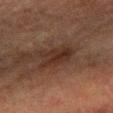notes — no biopsy performed (imaged during a skin exam)
size — about 4 mm
lighting — cross-polarized illumination
site — the right forearm
subject — female, aged 53–57
image — ~15 mm tile from a whole-body skin photo